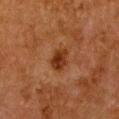Findings:
– follow-up: no biopsy performed (imaged during a skin exam)
– image source: 15 mm crop, total-body photography
– site: the chest
– subject: female, roughly 50 years of age
– size: about 3 mm
– illumination: cross-polarized illumination